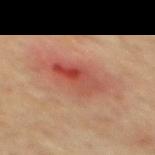The lesion-visualizer software estimated a footprint of about 10 mm² and a shape-asymmetry score of about 0.25 (0 = symmetric). The analysis additionally found a classifier nevus-likeness of about 0/100. A male subject, approximately 65 years of age. The tile uses cross-polarized illumination. A lesion tile, about 15 mm wide, cut from a 3D total-body photograph. On the mid back. The recorded lesion diameter is about 5 mm.